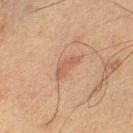Impression:
The lesion was photographed on a routine skin check and not biopsied; there is no pathology result.
Image and clinical context:
A male patient roughly 60 years of age. A roughly 15 mm field-of-view crop from a total-body skin photograph. Approximately 3.5 mm at its widest. Automated tile analysis of the lesion measured an average lesion color of about L≈44 a*≈18 b*≈25 (CIELAB), a lesion–skin lightness drop of about 6, and a lesion-to-skin contrast of about 5.5 (normalized; higher = more distinct). Located on the left lower leg.